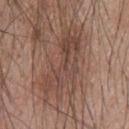Captured during whole-body skin photography for melanoma surveillance; the lesion was not biopsied. Cropped from a whole-body photographic skin survey; the tile spans about 15 mm. Measured at roughly 9.5 mm in maximum diameter. The subject is a male roughly 45 years of age. The tile uses white-light illumination. The lesion is located on the upper back.